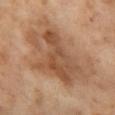Imaged during a routine full-body skin examination; the lesion was not biopsied and no histopathology is available. About 10 mm across. A 15 mm close-up tile from a total-body photography series done for melanoma screening. A female patient aged 53–57. The lesion is on the leg.